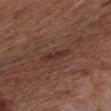The lesion was photographed on a routine skin check and not biopsied; there is no pathology result.
Captured under white-light illumination.
A 15 mm close-up tile from a total-body photography series done for melanoma screening.
The subject is a male aged 48–52.
The lesion-visualizer software estimated an average lesion color of about L≈32 a*≈20 b*≈23 (CIELAB) and a lesion-to-skin contrast of about 7 (normalized; higher = more distinct). The analysis additionally found a within-lesion color-variation index near 0.5/10 and radial color variation of about 0.
On the chest.
The lesion's longest dimension is about 3.5 mm.